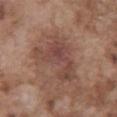Impression: Imaged during a routine full-body skin examination; the lesion was not biopsied and no histopathology is available. Image and clinical context: The lesion is on the chest. Automated tile analysis of the lesion measured a footprint of about 18 mm² and a shape-asymmetry score of about 0.55 (0 = symmetric). The software also gave a border-irregularity rating of about 7/10 and peripheral color asymmetry of about 1.5. This is a white-light tile. Measured at roughly 6.5 mm in maximum diameter. The subject is a male aged approximately 75. A 15 mm crop from a total-body photograph taken for skin-cancer surveillance.